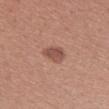Q: Was a biopsy performed?
A: total-body-photography surveillance lesion; no biopsy
Q: What is the anatomic site?
A: the arm
Q: What are the patient's age and sex?
A: female, aged 43–47
Q: What kind of image is this?
A: ~15 mm tile from a whole-body skin photo